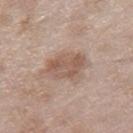This lesion was catalogued during total-body skin photography and was not selected for biopsy.
On the leg.
A close-up tile cropped from a whole-body skin photograph, about 15 mm across.
An algorithmic analysis of the crop reported a lesion area of about 11 mm², a shape eccentricity near 0.75, and a shape-asymmetry score of about 0.2 (0 = symmetric). The software also gave a nevus-likeness score of about 5/100 and lesion-presence confidence of about 100/100.
The subject is a male aged around 60.
The recorded lesion diameter is about 4.5 mm.